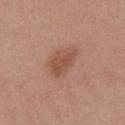Assessment:
No biopsy was performed on this lesion — it was imaged during a full skin examination and was not determined to be concerning.
Context:
On the chest. A female subject about 55 years old. An algorithmic analysis of the crop reported an area of roughly 8 mm², an eccentricity of roughly 0.75, and a symmetry-axis asymmetry near 0.25. And it measured border irregularity of about 2.5 on a 0–10 scale and radial color variation of about 1. The software also gave a classifier nevus-likeness of about 60/100 and a detector confidence of about 100 out of 100 that the crop contains a lesion. Captured under white-light illumination. Approximately 4 mm at its widest. A 15 mm crop from a total-body photograph taken for skin-cancer surveillance.